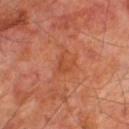Part of a total-body skin-imaging series; this lesion was reviewed on a skin check and was not flagged for biopsy. A male patient, approximately 70 years of age. A region of skin cropped from a whole-body photographic capture, roughly 15 mm wide. The lesion is on the right thigh.